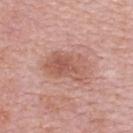biopsy_status: not biopsied; imaged during a skin examination
patient:
  sex: female
  age_approx: 65
image:
  source: total-body photography crop
  field_of_view_mm: 15
lighting: white-light
site: upper back
automated_metrics:
  area_mm2_approx: 13.0
  cielab_L: 56
  cielab_a: 24
  cielab_b: 28
  vs_skin_contrast_norm: 6.5
  border_irregularity_0_10: 3.5
  color_variation_0_10: 4.0
  peripheral_color_asymmetry: 1.5
  nevus_likeness_0_100: 15